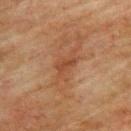Notes:
• follow-up: no biopsy performed (imaged during a skin exam)
• image source: ~15 mm crop, total-body skin-cancer survey
• size: about 2.5 mm
• location: the upper back
• patient: male, in their mid-70s
• lighting: cross-polarized